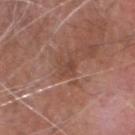biopsy_status: not biopsied; imaged during a skin examination
site: head or neck
patient:
  sex: male
  age_approx: 75
image:
  source: total-body photography crop
  field_of_view_mm: 15
automated_metrics:
  area_mm2_approx: 4.0
  eccentricity: 0.65
  cielab_L: 45
  cielab_a: 21
  cielab_b: 26
  vs_skin_contrast_norm: 5.5
  border_irregularity_0_10: 3.0
  color_variation_0_10: 2.5
  peripheral_color_asymmetry: 1.0